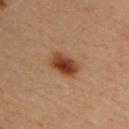Part of a total-body skin-imaging series; this lesion was reviewed on a skin check and was not flagged for biopsy.
Automated tile analysis of the lesion measured an area of roughly 7.5 mm². The software also gave a lesion color around L≈43 a*≈24 b*≈33 in CIELAB, a lesion–skin lightness drop of about 14, and a normalized border contrast of about 11. It also reported a border-irregularity rating of about 1.5/10, a within-lesion color-variation index near 6/10, and a peripheral color-asymmetry measure near 1.5. The software also gave an automated nevus-likeness rating near 100 out of 100 and a lesion-detection confidence of about 100/100.
Captured under cross-polarized illumination.
Approximately 4 mm at its widest.
From the upper back.
This image is a 15 mm lesion crop taken from a total-body photograph.
A female patient aged 33 to 37.